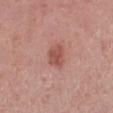The lesion was photographed on a routine skin check and not biopsied; there is no pathology result. Measured at roughly 3 mm in maximum diameter. A close-up tile cropped from a whole-body skin photograph, about 15 mm across. A male patient roughly 70 years of age. From the leg.